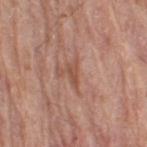Captured during whole-body skin photography for melanoma surveillance; the lesion was not biopsied. Longest diameter approximately 2.5 mm. The subject is a male roughly 80 years of age. Captured under white-light illumination. On the right thigh. This image is a 15 mm lesion crop taken from a total-body photograph.